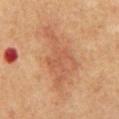{
  "patient": {
    "sex": "male",
    "age_approx": 60
  },
  "lesion_size": {
    "long_diameter_mm_approx": 9.0
  },
  "lighting": "cross-polarized",
  "image": {
    "source": "total-body photography crop",
    "field_of_view_mm": 15
  },
  "automated_metrics": {
    "area_mm2_approx": 25.0,
    "shape_asymmetry": 0.4,
    "vs_skin_darker_L": 7.0,
    "vs_skin_contrast_norm": 5.5,
    "border_irregularity_0_10": 7.0,
    "color_variation_0_10": 3.0,
    "peripheral_color_asymmetry": 1.0,
    "nevus_likeness_0_100": 5,
    "lesion_detection_confidence_0_100": 100
  },
  "site": "mid back"
}